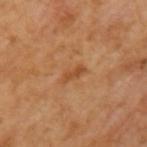– patient: male, approximately 65 years of age
– size: ~3 mm (longest diameter)
– image: total-body-photography crop, ~15 mm field of view
– automated lesion analysis: a classifier nevus-likeness of about 0/100